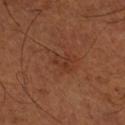| field | value |
|---|---|
| follow-up | no biopsy performed (imaged during a skin exam) |